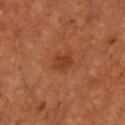Impression:
No biopsy was performed on this lesion — it was imaged during a full skin examination and was not determined to be concerning.
Image and clinical context:
The lesion is located on the chest. Captured under cross-polarized illumination. Longest diameter approximately 3 mm. A lesion tile, about 15 mm wide, cut from a 3D total-body photograph. A male subject approximately 75 years of age. Automated image analysis of the tile measured a footprint of about 5 mm², an outline eccentricity of about 0.65 (0 = round, 1 = elongated), and a shape-asymmetry score of about 0.25 (0 = symmetric). The analysis additionally found a border-irregularity index near 2.5/10, a within-lesion color-variation index near 1.5/10, and radial color variation of about 0.5.